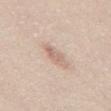A 15 mm close-up extracted from a 3D total-body photography capture.
The lesion is located on the front of the torso.
Captured under white-light illumination.
Measured at roughly 3.5 mm in maximum diameter.
A male patient aged 43 to 47.